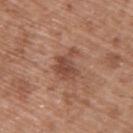| key | value |
|---|---|
| biopsy status | total-body-photography surveillance lesion; no biopsy |
| tile lighting | white-light illumination |
| acquisition | ~15 mm crop, total-body skin-cancer survey |
| location | the upper back |
| patient | male, roughly 50 years of age |
| diameter | ≈3.5 mm |